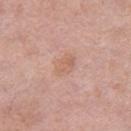Assessment: This lesion was catalogued during total-body skin photography and was not selected for biopsy. Image and clinical context: From the leg. Captured under white-light illumination. The subject is a female aged approximately 40. A 15 mm close-up tile from a total-body photography series done for melanoma screening.